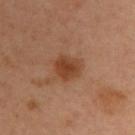  biopsy_status: not biopsied; imaged during a skin examination
  lesion_size:
    long_diameter_mm_approx: 3.5
  automated_metrics:
    nevus_likeness_0_100: 85
    lesion_detection_confidence_0_100: 100
  lighting: cross-polarized
  image:
    source: total-body photography crop
    field_of_view_mm: 15
  patient:
    sex: male
    age_approx: 40
  site: upper back